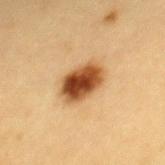biopsy_status: not biopsied; imaged during a skin examination
patient:
  sex: female
  age_approx: 30
site: upper back
image:
  source: total-body photography crop
  field_of_view_mm: 15